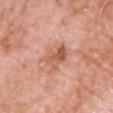This lesion was catalogued during total-body skin photography and was not selected for biopsy.
The lesion-visualizer software estimated a footprint of about 7 mm², an outline eccentricity of about 0.55 (0 = round, 1 = elongated), and two-axis asymmetry of about 0.25. The software also gave a mean CIELAB color near L≈58 a*≈25 b*≈32. And it measured a detector confidence of about 100 out of 100 that the crop contains a lesion.
Cropped from a total-body skin-imaging series; the visible field is about 15 mm.
A male patient approximately 60 years of age.
Captured under white-light illumination.
On the chest.